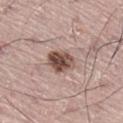Captured during whole-body skin photography for melanoma surveillance; the lesion was not biopsied. A male subject approximately 70 years of age. Automated image analysis of the tile measured a mean CIELAB color near L≈49 a*≈18 b*≈23, roughly 16 lightness units darker than nearby skin, and a normalized border contrast of about 11.5. Imaged with white-light lighting. Located on the leg. The recorded lesion diameter is about 3.5 mm. A region of skin cropped from a whole-body photographic capture, roughly 15 mm wide.